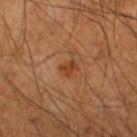| feature | finding |
|---|---|
| notes | total-body-photography surveillance lesion; no biopsy |
| image-analysis metrics | an outline eccentricity of about 0.7 (0 = round, 1 = elongated) and a symmetry-axis asymmetry near 0.45; a mean CIELAB color near L≈38 a*≈24 b*≈34 and a normalized lesion–skin contrast near 7.5 |
| patient | male, aged 43 to 47 |
| location | the left lower leg |
| image source | ~15 mm crop, total-body skin-cancer survey |
| lighting | cross-polarized illumination |